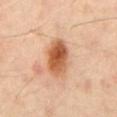biopsy_status: not biopsied; imaged during a skin examination
patient:
  sex: male
  age_approx: 40
image:
  source: total-body photography crop
  field_of_view_mm: 15
site: back